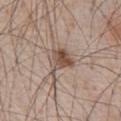Q: Automated lesion metrics?
A: an automated nevus-likeness rating near 100 out of 100 and lesion-presence confidence of about 100/100
Q: What is the anatomic site?
A: the chest
Q: How was the tile lit?
A: white-light
Q: What are the patient's age and sex?
A: male, about 65 years old
Q: What is the imaging modality?
A: total-body-photography crop, ~15 mm field of view
Q: Lesion size?
A: ~3.5 mm (longest diameter)